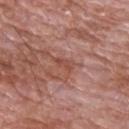patient:
  sex: male
  age_approx: 60
site: upper back
lighting: white-light
image:
  source: total-body photography crop
  field_of_view_mm: 15
lesion_size:
  long_diameter_mm_approx: 2.5
automated_metrics:
  area_mm2_approx: 2.5
  shape_asymmetry: 0.4
  cielab_L: 48
  cielab_a: 25
  cielab_b: 27
  vs_skin_darker_L: 7.0
  vs_skin_contrast_norm: 5.5